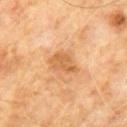Q: Is there a histopathology result?
A: total-body-photography surveillance lesion; no biopsy
Q: What is the anatomic site?
A: the abdomen
Q: Illumination type?
A: cross-polarized illumination
Q: What kind of image is this?
A: ~15 mm crop, total-body skin-cancer survey
Q: Automated lesion metrics?
A: a footprint of about 6.5 mm², an outline eccentricity of about 0.75 (0 = round, 1 = elongated), and a shape-asymmetry score of about 0.25 (0 = symmetric)
Q: What are the patient's age and sex?
A: male, about 60 years old